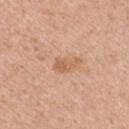<lesion>
  <biopsy_status>not biopsied; imaged during a skin examination</biopsy_status>
  <lesion_size>
    <long_diameter_mm_approx>3.0</long_diameter_mm_approx>
  </lesion_size>
  <lighting>white-light</lighting>
  <patient>
    <sex>male</sex>
    <age_approx>45</age_approx>
  </patient>
  <site>arm</site>
  <image>
    <source>total-body photography crop</source>
    <field_of_view_mm>15</field_of_view_mm>
  </image>
</lesion>